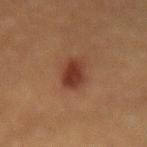Findings:
• biopsy status · imaged on a skin check; not biopsied
• size · about 4 mm
• TBP lesion metrics · an area of roughly 8 mm², an eccentricity of roughly 0.85, and a shape-asymmetry score of about 0.15 (0 = symmetric)
• subject · male, roughly 50 years of age
• imaging modality · 15 mm crop, total-body photography
• illumination · cross-polarized illumination
• site · the lower back